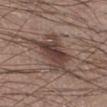Captured during whole-body skin photography for melanoma surveillance; the lesion was not biopsied. Measured at roughly 8 mm in maximum diameter. An algorithmic analysis of the crop reported a shape eccentricity near 0.8 and two-axis asymmetry of about 0.45. The software also gave a mean CIELAB color near L≈42 a*≈16 b*≈21, roughly 11 lightness units darker than nearby skin, and a normalized lesion–skin contrast near 9. The software also gave border irregularity of about 7 on a 0–10 scale, a color-variation rating of about 6/10, and peripheral color asymmetry of about 2. A 15 mm close-up extracted from a 3D total-body photography capture. A male patient aged approximately 20. On the right lower leg. Captured under white-light illumination.